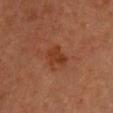acquisition: ~15 mm tile from a whole-body skin photo | TBP lesion metrics: a color-variation rating of about 2/10; an automated nevus-likeness rating near 10 out of 100 and a detector confidence of about 100 out of 100 that the crop contains a lesion | body site: the front of the torso | patient: female, about 40 years old | illumination: cross-polarized.